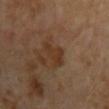biopsy status: total-body-photography surveillance lesion; no biopsy | size: ~3.5 mm (longest diameter) | illumination: cross-polarized | patient: male, aged 63–67 | imaging modality: ~15 mm tile from a whole-body skin photo | anatomic site: the right upper arm.